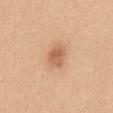Captured under white-light illumination.
A male subject, approximately 50 years of age.
On the abdomen.
A 15 mm close-up tile from a total-body photography series done for melanoma screening.
Approximately 3 mm at its widest.